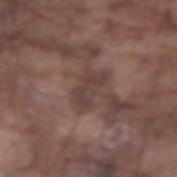– biopsy status: no biopsy performed (imaged during a skin exam)
– illumination: white-light
– imaging modality: ~15 mm tile from a whole-body skin photo
– body site: the arm
– subject: male, aged approximately 75